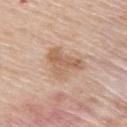No biopsy was performed on this lesion — it was imaged during a full skin examination and was not determined to be concerning. Imaged with white-light lighting. A 15 mm close-up tile from a total-body photography series done for melanoma screening. Located on the upper back. An algorithmic analysis of the crop reported an average lesion color of about L≈61 a*≈18 b*≈32 (CIELAB) and a lesion-to-skin contrast of about 7 (normalized; higher = more distinct). The software also gave a nevus-likeness score of about 0/100 and a lesion-detection confidence of about 100/100. A male subject, aged approximately 60. Longest diameter approximately 5 mm.